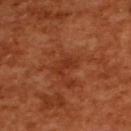Imaged during a routine full-body skin examination; the lesion was not biopsied and no histopathology is available.
The subject is a female aged 53 to 57.
Located on the upper back.
A lesion tile, about 15 mm wide, cut from a 3D total-body photograph.
This is a cross-polarized tile.
Longest diameter approximately 2.5 mm.
Automated image analysis of the tile measured a footprint of about 3 mm² and an eccentricity of roughly 0.85. The software also gave a lesion color around L≈36 a*≈30 b*≈36 in CIELAB and a normalized lesion–skin contrast near 5.5. The analysis additionally found a border-irregularity index near 5/10 and internal color variation of about 0 on a 0–10 scale.